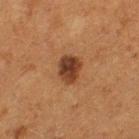Q: Is there a histopathology result?
A: total-body-photography surveillance lesion; no biopsy
Q: What is the imaging modality?
A: ~15 mm crop, total-body skin-cancer survey
Q: Lesion size?
A: ≈3.5 mm
Q: Patient demographics?
A: female, aged 48–52
Q: What is the anatomic site?
A: the left thigh
Q: Illumination type?
A: cross-polarized illumination